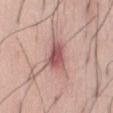Part of a total-body skin-imaging series; this lesion was reviewed on a skin check and was not flagged for biopsy. The patient is a male aged 33 to 37. The lesion's longest dimension is about 5.5 mm. From the abdomen. A close-up tile cropped from a whole-body skin photograph, about 15 mm across. This is a white-light tile. The total-body-photography lesion software estimated a lesion area of about 11 mm², a shape eccentricity near 0.85, and two-axis asymmetry of about 0.4. The analysis additionally found a lesion color around L≈57 a*≈23 b*≈22 in CIELAB, a lesion–skin lightness drop of about 12, and a normalized border contrast of about 8. It also reported a classifier nevus-likeness of about 25/100 and lesion-presence confidence of about 100/100.